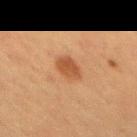follow-up: total-body-photography surveillance lesion; no biopsy | automated metrics: a lesion–skin lightness drop of about 9 and a normalized border contrast of about 7.5 | anatomic site: the mid back | tile lighting: cross-polarized illumination | size: ≈2.5 mm | acquisition: total-body-photography crop, ~15 mm field of view | patient: female, in their mid- to late 50s.